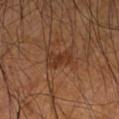notes — total-body-photography surveillance lesion; no biopsy.